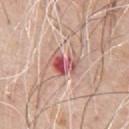workup = catalogued during a skin exam; not biopsied
lesion diameter = about 4 mm
image source = total-body-photography crop, ~15 mm field of view
automated lesion analysis = a classifier nevus-likeness of about 0/100 and a lesion-detection confidence of about 100/100
tile lighting = white-light illumination
site = the chest
subject = male, in their 70s